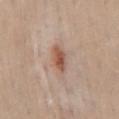An algorithmic analysis of the crop reported a mean CIELAB color near L≈54 a*≈21 b*≈29, a lesion–skin lightness drop of about 12, and a normalized border contrast of about 8.5. The analysis additionally found a within-lesion color-variation index near 4/10 and peripheral color asymmetry of about 1.
A male subject aged around 40.
The recorded lesion diameter is about 3.5 mm.
The lesion is located on the mid back.
Imaged with white-light lighting.
Cropped from a total-body skin-imaging series; the visible field is about 15 mm.